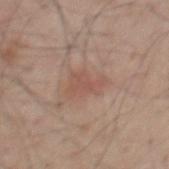The lesion was tiled from a total-body skin photograph and was not biopsied. Longest diameter approximately 4 mm. Captured under white-light illumination. Located on the mid back. A male patient, aged around 50. Cropped from a total-body skin-imaging series; the visible field is about 15 mm.